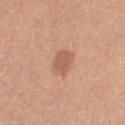follow-up = no biopsy performed (imaged during a skin exam)
acquisition = ~15 mm tile from a whole-body skin photo
subject = female, aged approximately 45
image-analysis metrics = a lesion color around L≈58 a*≈23 b*≈32 in CIELAB and roughly 9 lightness units darker than nearby skin; a border-irregularity rating of about 2/10, a color-variation rating of about 1.5/10, and peripheral color asymmetry of about 0.5; a detector confidence of about 100 out of 100 that the crop contains a lesion
body site = the leg
lesion size = ≈3 mm
lighting = white-light illumination